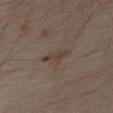Clinical impression:
This lesion was catalogued during total-body skin photography and was not selected for biopsy.